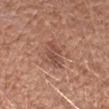<case>
<biopsy_status>not biopsied; imaged during a skin examination</biopsy_status>
<patient>
  <sex>male</sex>
  <age_approx>50</age_approx>
</patient>
<image>
  <source>total-body photography crop</source>
  <field_of_view_mm>15</field_of_view_mm>
</image>
<site>right upper arm</site>
<automated_metrics>
  <area_mm2_approx>5.5</area_mm2_approx>
  <eccentricity>0.85</eccentricity>
  <shape_asymmetry>0.3</shape_asymmetry>
  <cielab_L>49</cielab_L>
  <cielab_a>23</cielab_a>
  <cielab_b>27</cielab_b>
  <vs_skin_darker_L>9.0</vs_skin_darker_L>
  <nevus_likeness_0_100>10</nevus_likeness_0_100>
  <lesion_detection_confidence_0_100>100</lesion_detection_confidence_0_100>
</automated_metrics>
</case>